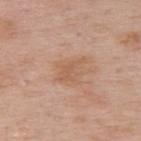Imaged during a routine full-body skin examination; the lesion was not biopsied and no histopathology is available.
A female patient about 50 years old.
Imaged with white-light lighting.
This image is a 15 mm lesion crop taken from a total-body photograph.
The lesion is on the upper back.
The total-body-photography lesion software estimated internal color variation of about 2.5 on a 0–10 scale and a peripheral color-asymmetry measure near 1. The software also gave lesion-presence confidence of about 100/100.
The recorded lesion diameter is about 4 mm.